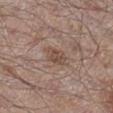notes: catalogued during a skin exam; not biopsied.